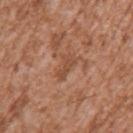follow-up: total-body-photography surveillance lesion; no biopsy | location: the left upper arm | TBP lesion metrics: an area of roughly 4 mm² and an eccentricity of roughly 0.9; a classifier nevus-likeness of about 0/100 and lesion-presence confidence of about 95/100 | size: ~3.5 mm (longest diameter) | image source: ~15 mm tile from a whole-body skin photo | subject: male, in their mid-40s.